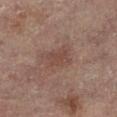Q: Was this lesion biopsied?
A: imaged on a skin check; not biopsied
Q: Who is the patient?
A: female, aged approximately 80
Q: What lighting was used for the tile?
A: cross-polarized
Q: What is the anatomic site?
A: the right leg
Q: How large is the lesion?
A: about 3 mm
Q: What is the imaging modality?
A: ~15 mm crop, total-body skin-cancer survey